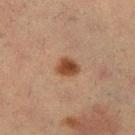workup — imaged on a skin check; not biopsied
image — total-body-photography crop, ~15 mm field of view
body site — the leg
subject — female, in their mid- to late 50s